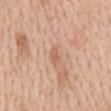Case summary:
– workup: imaged on a skin check; not biopsied
– imaging modality: ~15 mm tile from a whole-body skin photo
– diameter: ~2.5 mm (longest diameter)
– automated lesion analysis: an average lesion color of about L≈61 a*≈21 b*≈32 (CIELAB) and about 8 CIELAB-L* units darker than the surrounding skin; border irregularity of about 3 on a 0–10 scale, a within-lesion color-variation index near 0/10, and peripheral color asymmetry of about 0
– subject: male, aged around 60
– illumination: white-light illumination
– site: the mid back